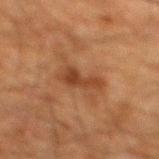Notes:
- biopsy status: no biopsy performed (imaged during a skin exam)
- body site: the mid back
- imaging modality: ~15 mm tile from a whole-body skin photo
- patient: male, aged 58 to 62